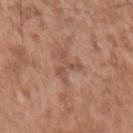Impression: Captured during whole-body skin photography for melanoma surveillance; the lesion was not biopsied. Clinical summary: The subject is a male aged 48–52. Located on the arm. About 3.5 mm across. This image is a 15 mm lesion crop taken from a total-body photograph.